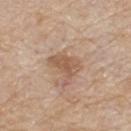The lesion was tiled from a total-body skin photograph and was not biopsied. A region of skin cropped from a whole-body photographic capture, roughly 15 mm wide. The recorded lesion diameter is about 3.5 mm. This is a white-light tile. Automated image analysis of the tile measured an area of roughly 6.5 mm², an eccentricity of roughly 0.8, and a symmetry-axis asymmetry near 0.35. The analysis additionally found a mean CIELAB color near L≈56 a*≈18 b*≈31. From the back. The subject is a male aged around 80.